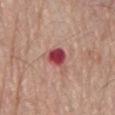Imaged during a routine full-body skin examination; the lesion was not biopsied and no histopathology is available. Automated tile analysis of the lesion measured an average lesion color of about L≈45 a*≈36 b*≈22 (CIELAB), a lesion–skin lightness drop of about 17, and a lesion-to-skin contrast of about 12.5 (normalized; higher = more distinct). And it measured a border-irregularity index near 2/10, a within-lesion color-variation index near 3.5/10, and peripheral color asymmetry of about 1. The software also gave lesion-presence confidence of about 100/100. Approximately 2.5 mm at its widest. This image is a 15 mm lesion crop taken from a total-body photograph. A male subject, aged around 80. The tile uses white-light illumination. The lesion is on the mid back.